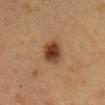Case summary:
- follow-up: imaged on a skin check; not biopsied
- site: the mid back
- automated lesion analysis: a lesion color around L≈36 a*≈20 b*≈30 in CIELAB, about 13 CIELAB-L* units darker than the surrounding skin, and a normalized lesion–skin contrast near 11
- lesion diameter: about 3.5 mm
- lighting: cross-polarized illumination
- image: total-body-photography crop, ~15 mm field of view
- subject: male, in their mid-50s